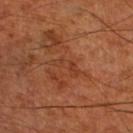Assessment: The lesion was tiled from a total-body skin photograph and was not biopsied. Image and clinical context: The lesion's longest dimension is about 3.5 mm. The subject is a male aged approximately 70. A 15 mm crop from a total-body photograph taken for skin-cancer surveillance. The lesion is located on the right thigh. Imaged with cross-polarized lighting.